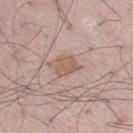The subject is a male about 55 years old.
This image is a 15 mm lesion crop taken from a total-body photograph.
Imaged with white-light lighting.
The recorded lesion diameter is about 3 mm.
From the right thigh.